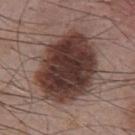No biopsy was performed on this lesion — it was imaged during a full skin examination and was not determined to be concerning.
This is a white-light tile.
The patient is a male roughly 55 years of age.
Automated image analysis of the tile measured an area of roughly 45 mm², a shape eccentricity near 0.7, and two-axis asymmetry of about 0.15. And it measured a mean CIELAB color near L≈36 a*≈17 b*≈20, a lesion–skin lightness drop of about 17, and a normalized border contrast of about 13.5. It also reported border irregularity of about 2 on a 0–10 scale and radial color variation of about 1.5.
From the front of the torso.
A region of skin cropped from a whole-body photographic capture, roughly 15 mm wide.